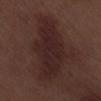Q: Is there a histopathology result?
A: imaged on a skin check; not biopsied
Q: What lighting was used for the tile?
A: white-light
Q: What did automated image analysis measure?
A: an area of roughly 36 mm², an eccentricity of roughly 0.8, and a shape-asymmetry score of about 0.35 (0 = symmetric); a mean CIELAB color near L≈24 a*≈18 b*≈17, about 7 CIELAB-L* units darker than the surrounding skin, and a lesion-to-skin contrast of about 8.5 (normalized; higher = more distinct); radial color variation of about 1; a nevus-likeness score of about 5/100 and lesion-presence confidence of about 100/100
Q: What are the patient's age and sex?
A: male, aged 68–72
Q: What is the lesion's diameter?
A: ≈9.5 mm
Q: What is the imaging modality?
A: ~15 mm tile from a whole-body skin photo
Q: Where on the body is the lesion?
A: the right lower leg